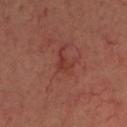| key | value |
|---|---|
| image-analysis metrics | a footprint of about 3 mm², a shape eccentricity near 0.8, and two-axis asymmetry of about 0.55 |
| patient | female, about 55 years old |
| image source | total-body-photography crop, ~15 mm field of view |
| body site | the head or neck |
| lesion size | ~2.5 mm (longest diameter) |